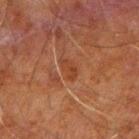{"biopsy_status": "not biopsied; imaged during a skin examination", "patient": {"sex": "male", "age_approx": 60}, "lesion_size": {"long_diameter_mm_approx": 2.5}, "lighting": "cross-polarized", "site": "left arm", "image": {"source": "total-body photography crop", "field_of_view_mm": 15}}